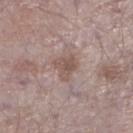Recorded during total-body skin imaging; not selected for excision or biopsy. Located on the right lower leg. A 15 mm crop from a total-body photograph taken for skin-cancer surveillance. A male subject, roughly 75 years of age.